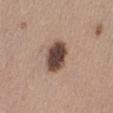workup: imaged on a skin check; not biopsied
tile lighting: white-light
site: the abdomen
diameter: about 4 mm
subject: female, aged approximately 50
automated lesion analysis: a footprint of about 10 mm², a shape eccentricity near 0.65, and a shape-asymmetry score of about 0.15 (0 = symmetric); radial color variation of about 1; a nevus-likeness score of about 40/100 and lesion-presence confidence of about 100/100
image source: 15 mm crop, total-body photography